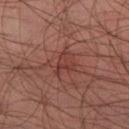| field | value |
|---|---|
| patient | male, roughly 45 years of age |
| lesion diameter | ≈3.5 mm |
| automated lesion analysis | an area of roughly 4 mm² and a symmetry-axis asymmetry near 0.65; a border-irregularity rating of about 7/10, a color-variation rating of about 2/10, and radial color variation of about 0.5; a classifier nevus-likeness of about 15/100 and lesion-presence confidence of about 95/100 |
| image source | 15 mm crop, total-body photography |
| anatomic site | the leg |